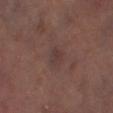Part of a total-body skin-imaging series; this lesion was reviewed on a skin check and was not flagged for biopsy.
A 15 mm crop from a total-body photograph taken for skin-cancer surveillance.
A male subject, in their mid-60s.
The total-body-photography lesion software estimated an eccentricity of roughly 0.8 and two-axis asymmetry of about 0.3. It also reported an automated nevus-likeness rating near 0 out of 100 and a lesion-detection confidence of about 95/100.
From the left lower leg.